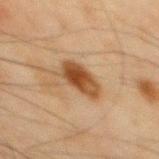Assessment:
Captured during whole-body skin photography for melanoma surveillance; the lesion was not biopsied.
Clinical summary:
A 15 mm close-up tile from a total-body photography series done for melanoma screening. A male patient in their mid-40s. From the back.